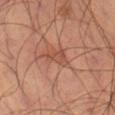The lesion was photographed on a routine skin check and not biopsied; there is no pathology result.
A region of skin cropped from a whole-body photographic capture, roughly 15 mm wide.
A male patient aged 63 to 67.
Located on the left thigh.
The lesion's longest dimension is about 3 mm.
Automated tile analysis of the lesion measured a footprint of about 4.5 mm², an eccentricity of roughly 0.75, and a symmetry-axis asymmetry near 0.45. The software also gave a lesion color around L≈47 a*≈24 b*≈29 in CIELAB, roughly 7 lightness units darker than nearby skin, and a normalized lesion–skin contrast near 5.5.
The tile uses cross-polarized illumination.